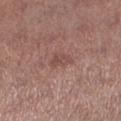Recorded during total-body skin imaging; not selected for excision or biopsy. The subject is a female aged approximately 50. Cropped from a whole-body photographic skin survey; the tile spans about 15 mm. Automated tile analysis of the lesion measured a mean CIELAB color near L≈48 a*≈21 b*≈23, about 7 CIELAB-L* units darker than the surrounding skin, and a normalized lesion–skin contrast near 5.5. Longest diameter approximately 3 mm. The lesion is located on the left lower leg.